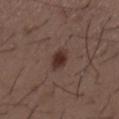Part of a total-body skin-imaging series; this lesion was reviewed on a skin check and was not flagged for biopsy.
The tile uses white-light illumination.
The lesion's longest dimension is about 3.5 mm.
A male subject, in their 50s.
On the back.
A lesion tile, about 15 mm wide, cut from a 3D total-body photograph.